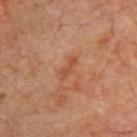| feature | finding |
|---|---|
| notes | no biopsy performed (imaged during a skin exam) |
| diameter | ≈3 mm |
| site | the front of the torso |
| image | total-body-photography crop, ~15 mm field of view |
| tile lighting | cross-polarized |
| subject | male, aged 63 to 67 |